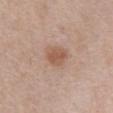<lesion>
<automated_metrics>
  <eccentricity>0.45</eccentricity>
  <border_irregularity_0_10>2.0</border_irregularity_0_10>
  <color_variation_0_10>2.0</color_variation_0_10>
  <peripheral_color_asymmetry>0.5</peripheral_color_asymmetry>
</automated_metrics>
<lesion_size>
  <long_diameter_mm_approx>3.0</long_diameter_mm_approx>
</lesion_size>
<patient>
  <sex>female</sex>
  <age_approx>60</age_approx>
</patient>
<image>
  <source>total-body photography crop</source>
  <field_of_view_mm>15</field_of_view_mm>
</image>
<site>abdomen</site>
<lighting>white-light</lighting>
</lesion>